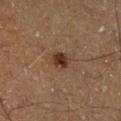Assessment:
Captured during whole-body skin photography for melanoma surveillance; the lesion was not biopsied.
Acquisition and patient details:
The tile uses cross-polarized illumination. Automated image analysis of the tile measured an area of roughly 3.5 mm², an eccentricity of roughly 0.5, and a symmetry-axis asymmetry near 0.25. It also reported an average lesion color of about L≈27 a*≈15 b*≈23 (CIELAB) and a normalized lesion–skin contrast near 10.5. It also reported an automated nevus-likeness rating near 90 out of 100 and a lesion-detection confidence of about 100/100. This image is a 15 mm lesion crop taken from a total-body photograph. The lesion is on the right lower leg. The subject is a male aged approximately 65.